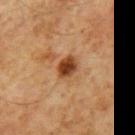Findings:
* workup: no biopsy performed (imaged during a skin exam)
* patient: male, aged 58 to 62
* acquisition: ~15 mm tile from a whole-body skin photo
* site: the mid back
* diameter: about 3 mm
* TBP lesion metrics: a footprint of about 5 mm², an outline eccentricity of about 0.7 (0 = round, 1 = elongated), and a shape-asymmetry score of about 0.15 (0 = symmetric); a mean CIELAB color near L≈39 a*≈23 b*≈34, roughly 15 lightness units darker than nearby skin, and a normalized border contrast of about 11.5; border irregularity of about 1.5 on a 0–10 scale, internal color variation of about 4 on a 0–10 scale, and peripheral color asymmetry of about 1; a nevus-likeness score of about 95/100
* illumination: cross-polarized